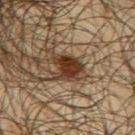The lesion was photographed on a routine skin check and not biopsied; there is no pathology result.
A 15 mm crop from a total-body photograph taken for skin-cancer surveillance.
The subject is a male aged approximately 65.
The lesion is on the upper back.
Imaged with cross-polarized lighting.